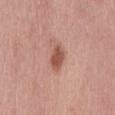<tbp_lesion>
<biopsy_status>not biopsied; imaged during a skin examination</biopsy_status>
<image>
  <source>total-body photography crop</source>
  <field_of_view_mm>15</field_of_view_mm>
</image>
<patient>
  <sex>male</sex>
  <age_approx>70</age_approx>
</patient>
<site>mid back</site>
<lesion_size>
  <long_diameter_mm_approx>3.5</long_diameter_mm_approx>
</lesion_size>
</tbp_lesion>